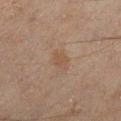The lesion was tiled from a total-body skin photograph and was not biopsied.
Approximately 3 mm at its widest.
On the left lower leg.
A 15 mm crop from a total-body photograph taken for skin-cancer surveillance.
A male patient, in their mid-40s.
Captured under cross-polarized illumination.